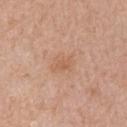workup: imaged on a skin check; not biopsied | site: the left arm | imaging modality: ~15 mm tile from a whole-body skin photo | patient: male, aged approximately 80 | TBP lesion metrics: a lesion color around L≈60 a*≈21 b*≈33 in CIELAB, about 6 CIELAB-L* units darker than the surrounding skin, and a normalized border contrast of about 5; a border-irregularity index near 3/10, a color-variation rating of about 1.5/10, and a peripheral color-asymmetry measure near 0.5; an automated nevus-likeness rating near 0 out of 100 and a lesion-detection confidence of about 100/100 | lighting: white-light illumination | lesion diameter: ~3 mm (longest diameter).